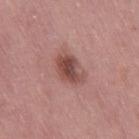| feature | finding |
|---|---|
| biopsy status | no biopsy performed (imaged during a skin exam) |
| anatomic site | the right thigh |
| subject | female, aged approximately 50 |
| TBP lesion metrics | a shape-asymmetry score of about 0.3 (0 = symmetric); an average lesion color of about L≈47 a*≈23 b*≈23 (CIELAB), roughly 12 lightness units darker than nearby skin, and a normalized lesion–skin contrast near 9; a border-irregularity index near 3/10, a within-lesion color-variation index near 4.5/10, and a peripheral color-asymmetry measure near 1.5 |
| lighting | white-light illumination |
| imaging modality | ~15 mm crop, total-body skin-cancer survey |